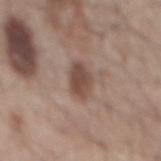Q: What is the lesion's diameter?
A: ≈3.5 mm
Q: What is the anatomic site?
A: the mid back
Q: What lighting was used for the tile?
A: white-light illumination
Q: How was this image acquired?
A: ~15 mm crop, total-body skin-cancer survey
Q: Automated lesion metrics?
A: a lesion color around L≈47 a*≈17 b*≈24 in CIELAB and about 11 CIELAB-L* units darker than the surrounding skin
Q: Patient demographics?
A: male, aged 53 to 57